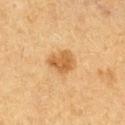Impression: Imaged during a routine full-body skin examination; the lesion was not biopsied and no histopathology is available.